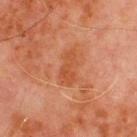{
  "biopsy_status": "not biopsied; imaged during a skin examination",
  "lesion_size": {
    "long_diameter_mm_approx": 4.0
  },
  "image": {
    "source": "total-body photography crop",
    "field_of_view_mm": 15
  },
  "patient": {
    "sex": "male",
    "age_approx": 70
  },
  "site": "chest",
  "lighting": "cross-polarized"
}